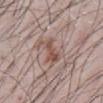Assessment: Part of a total-body skin-imaging series; this lesion was reviewed on a skin check and was not flagged for biopsy. Context: Imaged with white-light lighting. A male subject in their mid-60s. On the abdomen. Measured at roughly 4.5 mm in maximum diameter. A 15 mm crop from a total-body photograph taken for skin-cancer surveillance.